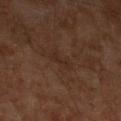A 15 mm close-up tile from a total-body photography series done for melanoma screening.
From the left lower leg.
Measured at roughly 2.5 mm in maximum diameter.
Automated image analysis of the tile measured an average lesion color of about L≈23 a*≈15 b*≈21 (CIELAB) and a normalized border contrast of about 5. And it measured an automated nevus-likeness rating near 0 out of 100 and a lesion-detection confidence of about 95/100.
A male subject aged 58–62.
The tile uses cross-polarized illumination.